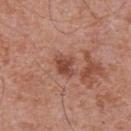Recorded during total-body skin imaging; not selected for excision or biopsy. On the chest. The tile uses white-light illumination. An algorithmic analysis of the crop reported a footprint of about 5 mm², an eccentricity of roughly 0.55, and a shape-asymmetry score of about 0.2 (0 = symmetric). The software also gave a lesion color around L≈47 a*≈25 b*≈28 in CIELAB, about 11 CIELAB-L* units darker than the surrounding skin, and a lesion-to-skin contrast of about 8 (normalized; higher = more distinct). And it measured border irregularity of about 2.5 on a 0–10 scale, a color-variation rating of about 2.5/10, and radial color variation of about 1. A 15 mm close-up tile from a total-body photography series done for melanoma screening. The patient is a male about 70 years old. Longest diameter approximately 2.5 mm.